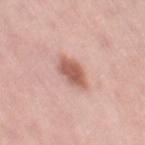biopsy status: catalogued during a skin exam; not biopsied | patient: female, aged 48–52 | location: the right thigh | image: ~15 mm crop, total-body skin-cancer survey | size: ≈4 mm.